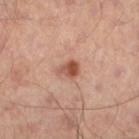Recorded during total-body skin imaging; not selected for excision or biopsy.